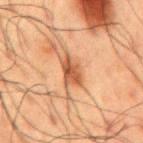No biopsy was performed on this lesion — it was imaged during a full skin examination and was not determined to be concerning. A male patient in their 60s. The lesion is located on the mid back. Cropped from a total-body skin-imaging series; the visible field is about 15 mm.